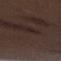biopsy_status: not biopsied; imaged during a skin examination
image:
  source: total-body photography crop
  field_of_view_mm: 15
lighting: white-light
lesion_size:
  long_diameter_mm_approx: 3.0
site: left forearm
patient:
  sex: male
  age_approx: 70